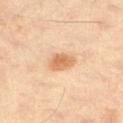Recorded during total-body skin imaging; not selected for excision or biopsy. A close-up tile cropped from a whole-body skin photograph, about 15 mm across. Located on the left thigh. The subject is a male roughly 60 years of age.